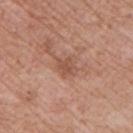image source: 15 mm crop, total-body photography | body site: the chest | diameter: ≈2.5 mm | image-analysis metrics: a footprint of about 4 mm² and two-axis asymmetry of about 0.3; a lesion color around L≈52 a*≈23 b*≈30 in CIELAB, roughly 8 lightness units darker than nearby skin, and a normalized border contrast of about 6 | tile lighting: white-light illumination | subject: male, aged approximately 65.